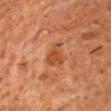This lesion was catalogued during total-body skin photography and was not selected for biopsy. A male subject aged approximately 65. Located on the chest. The tile uses cross-polarized illumination. A close-up tile cropped from a whole-body skin photograph, about 15 mm across. About 3 mm across.